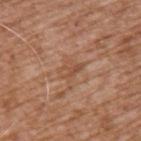Assessment:
Recorded during total-body skin imaging; not selected for excision or biopsy.
Clinical summary:
The patient is a male roughly 75 years of age. The lesion is located on the upper back. Measured at roughly 3 mm in maximum diameter. The tile uses white-light illumination. Cropped from a whole-body photographic skin survey; the tile spans about 15 mm.